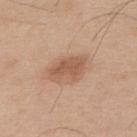Case summary:
- notes · imaged on a skin check; not biopsied
- patient · male, aged around 55
- TBP lesion metrics · a detector confidence of about 100 out of 100 that the crop contains a lesion
- image · 15 mm crop, total-body photography
- size · about 4 mm
- lighting · white-light illumination
- anatomic site · the back